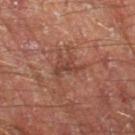The lesion was photographed on a routine skin check and not biopsied; there is no pathology result.
The patient is a male in their 70s.
Captured under cross-polarized illumination.
A region of skin cropped from a whole-body photographic capture, roughly 15 mm wide.
Approximately 3.5 mm at its widest.
On the left thigh.
The lesion-visualizer software estimated a footprint of about 4 mm² and a symmetry-axis asymmetry near 0.55. And it measured a lesion–skin lightness drop of about 7 and a normalized border contrast of about 6.